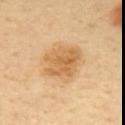The lesion was tiled from a total-body skin photograph and was not biopsied. Cropped from a total-body skin-imaging series; the visible field is about 15 mm. A female subject, aged 38 to 42. From the upper back.